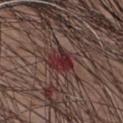This lesion was catalogued during total-body skin photography and was not selected for biopsy. About 3 mm across. Cropped from a total-body skin-imaging series; the visible field is about 15 mm. The patient is a male roughly 60 years of age. Automated tile analysis of the lesion measured a classifier nevus-likeness of about 0/100 and a lesion-detection confidence of about 100/100. On the chest.